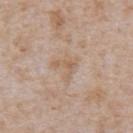Impression:
This lesion was catalogued during total-body skin photography and was not selected for biopsy.
Acquisition and patient details:
From the abdomen. Captured under white-light illumination. A lesion tile, about 15 mm wide, cut from a 3D total-body photograph. Approximately 2.5 mm at its widest. A male patient, aged around 65.